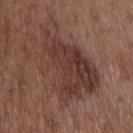No biopsy was performed on this lesion — it was imaged during a full skin examination and was not determined to be concerning. Captured under white-light illumination. Longest diameter approximately 9.5 mm. Located on the upper back. A roughly 15 mm field-of-view crop from a total-body skin photograph. A male subject aged approximately 50.